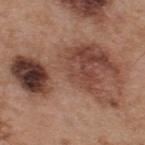Recorded during total-body skin imaging; not selected for excision or biopsy. The lesion is on the back. Cropped from a whole-body photographic skin survey; the tile spans about 15 mm. A male subject, approximately 55 years of age. Automated tile analysis of the lesion measured an average lesion color of about L≈44 a*≈21 b*≈26 (CIELAB). It also reported a border-irregularity index near 7/10, a within-lesion color-variation index near 10/10, and peripheral color asymmetry of about 4.5. The tile uses white-light illumination. About 12.5 mm across.